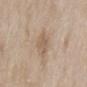Impression:
This lesion was catalogued during total-body skin photography and was not selected for biopsy.
Acquisition and patient details:
The lesion is located on the back. A 15 mm close-up extracted from a 3D total-body photography capture. The subject is a female aged 58 to 62.